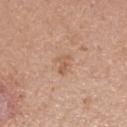Q: Was a biopsy performed?
A: catalogued during a skin exam; not biopsied
Q: Lesion size?
A: ≈2.5 mm
Q: Lesion location?
A: the right forearm
Q: What did automated image analysis measure?
A: a footprint of about 2.5 mm², a shape eccentricity near 0.9, and a symmetry-axis asymmetry near 0.5
Q: Patient demographics?
A: female, aged approximately 35
Q: What lighting was used for the tile?
A: white-light
Q: How was this image acquired?
A: ~15 mm tile from a whole-body skin photo